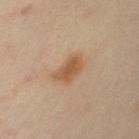Recorded during total-body skin imaging; not selected for excision or biopsy. From the left upper arm. A female patient, aged approximately 45. Cropped from a total-body skin-imaging series; the visible field is about 15 mm. Captured under cross-polarized illumination.